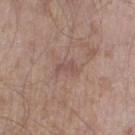Notes:
– notes: catalogued during a skin exam; not biopsied
– patient: male, aged approximately 45
– body site: the left thigh
– imaging modality: 15 mm crop, total-body photography